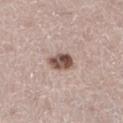biopsy status=no biopsy performed (imaged during a skin exam)
site=the left lower leg
subject=female, in their 40s
lesion diameter=about 3.5 mm
lighting=white-light
imaging modality=total-body-photography crop, ~15 mm field of view